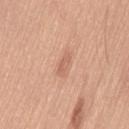Findings:
- follow-up — total-body-photography surveillance lesion; no biopsy
- image — total-body-photography crop, ~15 mm field of view
- lesion diameter — ≈3 mm
- site — the leg
- illumination — white-light
- patient — male, approximately 35 years of age